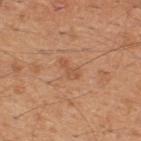  biopsy_status: not biopsied; imaged during a skin examination
  image:
    source: total-body photography crop
    field_of_view_mm: 15
  patient:
    sex: male
    age_approx: 45
  site: upper back